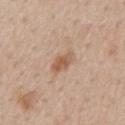The lesion was tiled from a total-body skin photograph and was not biopsied. A male subject, aged 58 to 62. Cropped from a total-body skin-imaging series; the visible field is about 15 mm. From the mid back.